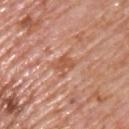  biopsy_status: not biopsied; imaged during a skin examination